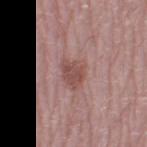follow-up: no biopsy performed (imaged during a skin exam); illumination: white-light illumination; site: the left thigh; size: about 3.5 mm; image source: 15 mm crop, total-body photography; subject: female, approximately 60 years of age.